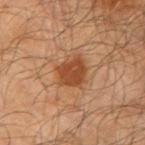Clinical impression: Imaged during a routine full-body skin examination; the lesion was not biopsied and no histopathology is available. Context: Longest diameter approximately 3.5 mm. The subject is a male aged approximately 65. On the right upper arm. Cropped from a whole-body photographic skin survey; the tile spans about 15 mm.